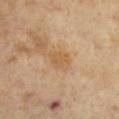{"biopsy_status": "not biopsied; imaged during a skin examination", "automated_metrics": {"area_mm2_approx": 4.5, "eccentricity": 0.65, "shape_asymmetry": 0.3, "cielab_L": 58, "cielab_a": 19, "cielab_b": 39, "vs_skin_darker_L": 7.0, "peripheral_color_asymmetry": 0.5, "nevus_likeness_0_100": 0}, "image": {"source": "total-body photography crop", "field_of_view_mm": 15}, "lighting": "cross-polarized", "site": "upper back", "lesion_size": {"long_diameter_mm_approx": 3.0}, "patient": {"sex": "female", "age_approx": 75}}